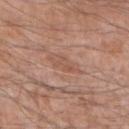<lesion>
<biopsy_status>not biopsied; imaged during a skin examination</biopsy_status>
<lesion_size>
  <long_diameter_mm_approx>2.5</long_diameter_mm_approx>
</lesion_size>
<lighting>white-light</lighting>
<automated_metrics>
  <cielab_L>53</cielab_L>
  <cielab_a>21</cielab_a>
  <cielab_b>29</cielab_b>
  <vs_skin_darker_L>6.0</vs_skin_darker_L>
  <vs_skin_contrast_norm>5.0</vs_skin_contrast_norm>
  <nevus_likeness_0_100>0</nevus_likeness_0_100>
</automated_metrics>
<patient>
  <sex>male</sex>
  <age_approx>60</age_approx>
</patient>
<image>
  <source>total-body photography crop</source>
  <field_of_view_mm>15</field_of_view_mm>
</image>
<site>right forearm</site>
</lesion>